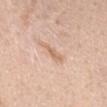biopsy status: imaged on a skin check; not biopsied | automated metrics: a lesion color around L≈66 a*≈19 b*≈32 in CIELAB and about 8 CIELAB-L* units darker than the surrounding skin; border irregularity of about 3 on a 0–10 scale, a color-variation rating of about 1.5/10, and peripheral color asymmetry of about 0.5 | lesion size: about 3 mm | tile lighting: white-light | subject: male, aged around 35 | location: the back | image source: total-body-photography crop, ~15 mm field of view.